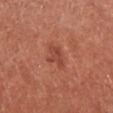Assessment: Part of a total-body skin-imaging series; this lesion was reviewed on a skin check and was not flagged for biopsy. Clinical summary: On the right lower leg. This is a white-light tile. A 15 mm crop from a total-body photograph taken for skin-cancer surveillance. Longest diameter approximately 3 mm. The total-body-photography lesion software estimated an average lesion color of about L≈46 a*≈29 b*≈31 (CIELAB), a lesion–skin lightness drop of about 8, and a lesion-to-skin contrast of about 6 (normalized; higher = more distinct). And it measured a classifier nevus-likeness of about 40/100 and a lesion-detection confidence of about 100/100. A male patient aged around 70.